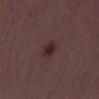notes — total-body-photography surveillance lesion; no biopsy | subject — male, approximately 50 years of age | diameter — ~2.5 mm (longest diameter) | imaging modality — ~15 mm crop, total-body skin-cancer survey | tile lighting — white-light illumination.